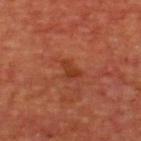Clinical impression: Recorded during total-body skin imaging; not selected for excision or biopsy. Acquisition and patient details: The lesion-visualizer software estimated a lesion area of about 3 mm², an outline eccentricity of about 0.8 (0 = round, 1 = elongated), and a symmetry-axis asymmetry near 0.5. The software also gave a border-irregularity rating of about 5/10 and radial color variation of about 0.5. And it measured a classifier nevus-likeness of about 0/100 and a detector confidence of about 100 out of 100 that the crop contains a lesion. Measured at roughly 2.5 mm in maximum diameter. The lesion is located on the upper back. A male subject aged 63–67. This is a cross-polarized tile. A lesion tile, about 15 mm wide, cut from a 3D total-body photograph.